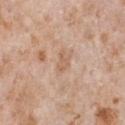Impression:
The lesion was photographed on a routine skin check and not biopsied; there is no pathology result.
Clinical summary:
A male patient about 65 years old. A roughly 15 mm field-of-view crop from a total-body skin photograph. From the chest.